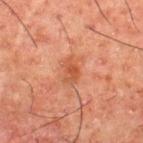Recorded during total-body skin imaging; not selected for excision or biopsy.
Imaged with cross-polarized lighting.
The subject is a male aged 48–52.
The lesion's longest dimension is about 3 mm.
Automated tile analysis of the lesion measured a lesion color around L≈42 a*≈24 b*≈30 in CIELAB and about 7 CIELAB-L* units darker than the surrounding skin. It also reported a classifier nevus-likeness of about 0/100 and a lesion-detection confidence of about 100/100.
A 15 mm close-up extracted from a 3D total-body photography capture.
The lesion is located on the upper back.